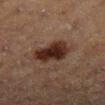The lesion was photographed on a routine skin check and not biopsied; there is no pathology result. The lesion is located on the left lower leg. A 15 mm close-up tile from a total-body photography series done for melanoma screening. Captured under cross-polarized illumination. A female patient in their 40s. Measured at roughly 4.5 mm in maximum diameter. The total-body-photography lesion software estimated a footprint of about 12 mm², a shape eccentricity near 0.6, and a shape-asymmetry score of about 0.25 (0 = symmetric). The software also gave a mean CIELAB color near L≈23 a*≈16 b*≈20, roughly 12 lightness units darker than nearby skin, and a normalized lesion–skin contrast near 13. And it measured a classifier nevus-likeness of about 95/100 and lesion-presence confidence of about 100/100.